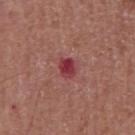  biopsy_status: not biopsied; imaged during a skin examination
  patient:
    sex: male
    age_approx: 65
  site: chest
  automated_metrics:
    area_mm2_approx: 4.5
    shape_asymmetry: 0.2
    cielab_L: 40
    cielab_a: 34
    cielab_b: 20
    vs_skin_darker_L: 11.0
    vs_skin_contrast_norm: 9.0
    border_irregularity_0_10: 2.0
    color_variation_0_10: 4.5
    peripheral_color_asymmetry: 1.5
    nevus_likeness_0_100: 0
  lighting: white-light
  image:
    source: total-body photography crop
    field_of_view_mm: 15
  lesion_size:
    long_diameter_mm_approx: 3.0